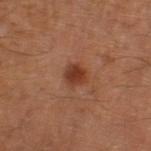Part of a total-body skin-imaging series; this lesion was reviewed on a skin check and was not flagged for biopsy.